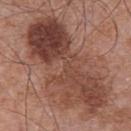image = 15 mm crop, total-body photography | anatomic site = the abdomen | subject = male, roughly 70 years of age.